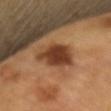Clinical summary:
A male patient aged 63 to 67. A 15 mm close-up extracted from a 3D total-body photography capture.